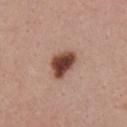Q: Where on the body is the lesion?
A: the chest
Q: How was this image acquired?
A: ~15 mm tile from a whole-body skin photo
Q: How large is the lesion?
A: about 4 mm
Q: How was the tile lit?
A: white-light illumination
Q: Patient demographics?
A: female, aged 23–27